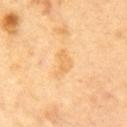Notes:
– tile lighting: cross-polarized
– subject: male, aged around 85 or older
– imaging modality: ~15 mm crop, total-body skin-cancer survey
– location: the chest